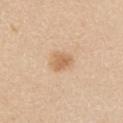No biopsy was performed on this lesion — it was imaged during a full skin examination and was not determined to be concerning.